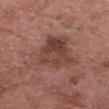– imaging modality: 15 mm crop, total-body photography
– subject: male, approximately 50 years of age
– size: about 7 mm
– illumination: white-light
– site: the head or neck